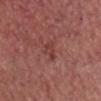diameter = about 2.5 mm | lighting = white-light | subject = male, aged 63 to 67 | imaging modality = ~15 mm crop, total-body skin-cancer survey | site = the chest.